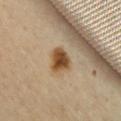biopsy status = total-body-photography surveillance lesion; no biopsy
subject = female, aged around 60
image source = ~15 mm crop, total-body skin-cancer survey
automated metrics = a border-irregularity index near 2.5/10, a within-lesion color-variation index near 4/10, and a peripheral color-asymmetry measure near 1
site = the chest
lighting = cross-polarized
lesion diameter = ≈3.5 mm